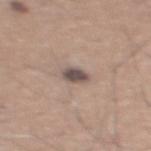Findings:
* follow-up · total-body-photography surveillance lesion; no biopsy
* subject · male, roughly 45 years of age
* acquisition · 15 mm crop, total-body photography
* anatomic site · the mid back